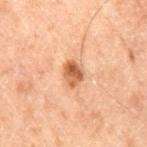Part of a total-body skin-imaging series; this lesion was reviewed on a skin check and was not flagged for biopsy. A 15 mm crop from a total-body photograph taken for skin-cancer surveillance. A male patient, aged approximately 70. On the right thigh.